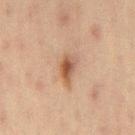Clinical impression: The lesion was tiled from a total-body skin photograph and was not biopsied. Context: A lesion tile, about 15 mm wide, cut from a 3D total-body photograph. The patient is a female aged around 45. About 3.5 mm across. Located on the left thigh. Captured under cross-polarized illumination. The total-body-photography lesion software estimated a nevus-likeness score of about 80/100 and a lesion-detection confidence of about 100/100.